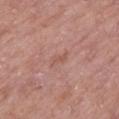| field | value |
|---|---|
| biopsy status | catalogued during a skin exam; not biopsied |
| patient | female, aged 73–77 |
| lesion diameter | ~2.5 mm (longest diameter) |
| tile lighting | white-light illumination |
| imaging modality | 15 mm crop, total-body photography |
| anatomic site | the left lower leg |